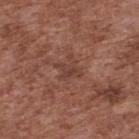{
  "biopsy_status": "not biopsied; imaged during a skin examination",
  "site": "upper back",
  "image": {
    "source": "total-body photography crop",
    "field_of_view_mm": 15
  },
  "patient": {
    "sex": "male",
    "age_approx": 75
  },
  "lesion_size": {
    "long_diameter_mm_approx": 3.0
  },
  "lighting": "white-light"
}